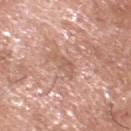Findings:
– workup · imaged on a skin check; not biopsied
– imaging modality · ~15 mm crop, total-body skin-cancer survey
– patient · male, about 65 years old
– anatomic site · the head or neck
– tile lighting · white-light
– lesion diameter · ~3 mm (longest diameter)